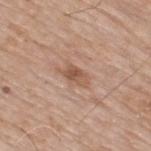Q: Is there a histopathology result?
A: no biopsy performed (imaged during a skin exam)
Q: What is the lesion's diameter?
A: ~3.5 mm (longest diameter)
Q: Automated lesion metrics?
A: an area of roughly 5 mm², a shape eccentricity near 0.85, and two-axis asymmetry of about 0.35; a lesion color around L≈55 a*≈20 b*≈30 in CIELAB, about 9 CIELAB-L* units darker than the surrounding skin, and a lesion-to-skin contrast of about 6.5 (normalized; higher = more distinct); internal color variation of about 4 on a 0–10 scale and peripheral color asymmetry of about 1.5; a detector confidence of about 100 out of 100 that the crop contains a lesion
Q: Patient demographics?
A: male, in their mid-60s
Q: How was the tile lit?
A: white-light
Q: How was this image acquired?
A: ~15 mm tile from a whole-body skin photo
Q: Lesion location?
A: the back